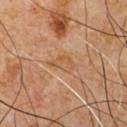The lesion was tiled from a total-body skin photograph and was not biopsied. A region of skin cropped from a whole-body photographic capture, roughly 15 mm wide. This is a cross-polarized tile. The lesion-visualizer software estimated a footprint of about 4 mm² and two-axis asymmetry of about 0.35. The analysis additionally found a lesion color around L≈53 a*≈21 b*≈35 in CIELAB and roughly 6 lightness units darker than nearby skin. The analysis additionally found a border-irregularity rating of about 3.5/10 and a color-variation rating of about 3/10. It also reported a nevus-likeness score of about 0/100 and a lesion-detection confidence of about 100/100. Located on the chest. The subject is a male approximately 60 years of age. Approximately 3 mm at its widest.